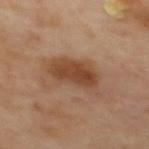<lesion>
  <biopsy_status>not biopsied; imaged during a skin examination</biopsy_status>
  <automated_metrics>
    <area_mm2_approx>15.0</area_mm2_approx>
    <shape_asymmetry>0.3</shape_asymmetry>
    <border_irregularity_0_10>3.5</border_irregularity_0_10>
    <color_variation_0_10>3.5</color_variation_0_10>
    <peripheral_color_asymmetry>1.5</peripheral_color_asymmetry>
    <nevus_likeness_0_100>90</nevus_likeness_0_100>
    <lesion_detection_confidence_0_100>100</lesion_detection_confidence_0_100>
  </automated_metrics>
  <site>mid back</site>
  <image>
    <source>total-body photography crop</source>
    <field_of_view_mm>15</field_of_view_mm>
  </image>
</lesion>